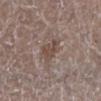Clinical impression: The lesion was tiled from a total-body skin photograph and was not biopsied. Context: Approximately 3 mm at its widest. From the leg. A roughly 15 mm field-of-view crop from a total-body skin photograph. This is a white-light tile. An algorithmic analysis of the crop reported an average lesion color of about L≈46 a*≈15 b*≈22 (CIELAB) and a lesion-to-skin contrast of about 7 (normalized; higher = more distinct). The analysis additionally found a border-irregularity index near 4/10, internal color variation of about 2 on a 0–10 scale, and radial color variation of about 0.5. The software also gave a classifier nevus-likeness of about 5/100 and a lesion-detection confidence of about 95/100. The patient is a male in their mid- to late 60s.